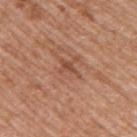The lesion was photographed on a routine skin check and not biopsied; there is no pathology result.
A 15 mm close-up tile from a total-body photography series done for melanoma screening.
Captured under white-light illumination.
A male patient, approximately 70 years of age.
Longest diameter approximately 3 mm.
The lesion is located on the upper back.